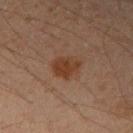Case summary:
- follow-up: catalogued during a skin exam; not biopsied
- acquisition: 15 mm crop, total-body photography
- subject: male, aged approximately 50
- TBP lesion metrics: a lesion area of about 6.5 mm² and a shape eccentricity near 0.65; an average lesion color of about L≈34 a*≈19 b*≈27 (CIELAB), a lesion–skin lightness drop of about 8, and a lesion-to-skin contrast of about 8 (normalized; higher = more distinct); a border-irregularity rating of about 2.5/10, internal color variation of about 2.5 on a 0–10 scale, and radial color variation of about 1
- size: ~3 mm (longest diameter)
- illumination: cross-polarized illumination
- location: the right upper arm